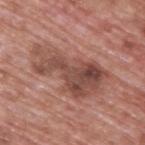Clinical impression: The lesion was tiled from a total-body skin photograph and was not biopsied. Background: A roughly 15 mm field-of-view crop from a total-body skin photograph. The subject is a male about 70 years old. Captured under white-light illumination. From the upper back.